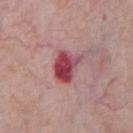Assessment:
Part of a total-body skin-imaging series; this lesion was reviewed on a skin check and was not flagged for biopsy.
Image and clinical context:
From the abdomen. The lesion-visualizer software estimated an area of roughly 9 mm², an outline eccentricity of about 0.7 (0 = round, 1 = elongated), and a symmetry-axis asymmetry near 0.25. A male patient about 60 years old. A close-up tile cropped from a whole-body skin photograph, about 15 mm across. The tile uses white-light illumination. Measured at roughly 4 mm in maximum diameter.